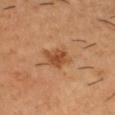Assessment: The lesion was tiled from a total-body skin photograph and was not biopsied. Background: A male patient, roughly 45 years of age. The lesion is on the head or neck. The recorded lesion diameter is about 3.5 mm. This image is a 15 mm lesion crop taken from a total-body photograph.